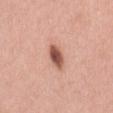No biopsy was performed on this lesion — it was imaged during a full skin examination and was not determined to be concerning. From the mid back. A male subject, aged approximately 60. Automated image analysis of the tile measured a lesion color around L≈54 a*≈25 b*≈28 in CIELAB, roughly 17 lightness units darker than nearby skin, and a lesion-to-skin contrast of about 11 (normalized; higher = more distinct). The analysis additionally found a border-irregularity index near 1.5/10, a color-variation rating of about 4/10, and peripheral color asymmetry of about 1. The software also gave an automated nevus-likeness rating near 100 out of 100. The tile uses white-light illumination. A roughly 15 mm field-of-view crop from a total-body skin photograph.